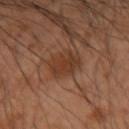Clinical summary:
A close-up tile cropped from a whole-body skin photograph, about 15 mm across. The lesion is located on the right forearm. The recorded lesion diameter is about 4 mm. A male patient, aged 53–57.